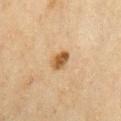Q: Is there a histopathology result?
A: imaged on a skin check; not biopsied
Q: Who is the patient?
A: male, roughly 70 years of age
Q: What kind of image is this?
A: 15 mm crop, total-body photography
Q: What did automated image analysis measure?
A: a lesion area of about 4.5 mm², a shape eccentricity near 0.65, and a shape-asymmetry score of about 0.2 (0 = symmetric); roughly 12 lightness units darker than nearby skin and a normalized border contrast of about 9.5; lesion-presence confidence of about 100/100
Q: What is the lesion's diameter?
A: ≈2.5 mm
Q: Where on the body is the lesion?
A: the right upper arm